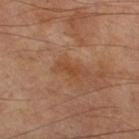Imaged during a routine full-body skin examination; the lesion was not biopsied and no histopathology is available.
This is a cross-polarized tile.
A male patient aged 68 to 72.
Cropped from a whole-body photographic skin survey; the tile spans about 15 mm.
Approximately 2.5 mm at its widest.
Automated image analysis of the tile measured a lesion area of about 3 mm², an eccentricity of roughly 0.9, and two-axis asymmetry of about 0.3. And it measured a nevus-likeness score of about 0/100 and a detector confidence of about 100 out of 100 that the crop contains a lesion.
Located on the leg.